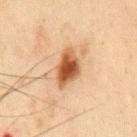Part of a total-body skin-imaging series; this lesion was reviewed on a skin check and was not flagged for biopsy. A male patient in their 60s. A 15 mm close-up extracted from a 3D total-body photography capture. This is a cross-polarized tile. Automated tile analysis of the lesion measured a mean CIELAB color near L≈47 a*≈21 b*≈33, a lesion–skin lightness drop of about 15, and a normalized border contrast of about 11. The lesion is located on the chest.